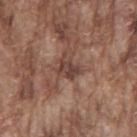Q: Was a biopsy performed?
A: catalogued during a skin exam; not biopsied